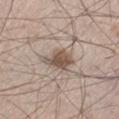Background: The subject is a male in their mid-40s. A close-up tile cropped from a whole-body skin photograph, about 15 mm across. Measured at roughly 4 mm in maximum diameter. On the leg. Captured under white-light illumination.